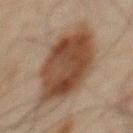The lesion was tiled from a total-body skin photograph and was not biopsied. A male subject in their mid- to late 40s. Captured under cross-polarized illumination. Located on the chest. A 15 mm close-up extracted from a 3D total-body photography capture.